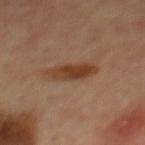Captured during whole-body skin photography for melanoma surveillance; the lesion was not biopsied.
A male patient, aged 63–67.
The lesion is located on the mid back.
Cropped from a total-body skin-imaging series; the visible field is about 15 mm.
The lesion's longest dimension is about 5 mm.
Captured under cross-polarized illumination.